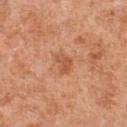biopsy status = no biopsy performed (imaged during a skin exam) | image source = 15 mm crop, total-body photography | location = the chest | lesion diameter = about 2.5 mm | illumination = white-light illumination | patient = male, about 55 years old.